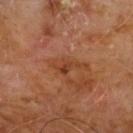workup — catalogued during a skin exam; not biopsied | site — the chest | automated lesion analysis — an area of roughly 3.5 mm² and a shape eccentricity near 0.85; a mean CIELAB color near L≈39 a*≈25 b*≈34, about 7 CIELAB-L* units darker than the surrounding skin, and a normalized lesion–skin contrast near 6.5 | subject — male, aged around 60 | size — ≈3.5 mm | image — ~15 mm crop, total-body skin-cancer survey.